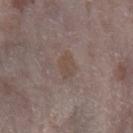Q: Was this lesion biopsied?
A: no biopsy performed (imaged during a skin exam)
Q: What is the imaging modality?
A: 15 mm crop, total-body photography
Q: Patient demographics?
A: female, aged approximately 65
Q: Where on the body is the lesion?
A: the left forearm